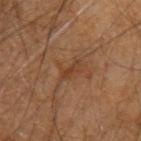Clinical impression:
Part of a total-body skin-imaging series; this lesion was reviewed on a skin check and was not flagged for biopsy.
Acquisition and patient details:
The lesion-visualizer software estimated a shape eccentricity near 0.95 and two-axis asymmetry of about 0.55. The lesion is on the left upper arm. The recorded lesion diameter is about 2.5 mm. A male patient, approximately 65 years of age. Cropped from a whole-body photographic skin survey; the tile spans about 15 mm. Imaged with cross-polarized lighting.